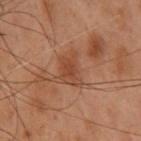Impression: Captured during whole-body skin photography for melanoma surveillance; the lesion was not biopsied. Context: A roughly 15 mm field-of-view crop from a total-body skin photograph. Located on the back. A female subject roughly 55 years of age. Measured at roughly 4 mm in maximum diameter.